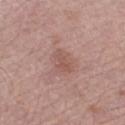biopsy status: imaged on a skin check; not biopsied
lesion size: about 3 mm
tile lighting: white-light illumination
image source: ~15 mm tile from a whole-body skin photo
body site: the left lower leg
automated lesion analysis: an outline eccentricity of about 0.85 (0 = round, 1 = elongated) and two-axis asymmetry of about 0.4; an average lesion color of about L≈53 a*≈22 b*≈24 (CIELAB), roughly 7 lightness units darker than nearby skin, and a normalized lesion–skin contrast near 5.5; border irregularity of about 4.5 on a 0–10 scale, internal color variation of about 1.5 on a 0–10 scale, and peripheral color asymmetry of about 0.5
subject: female, approximately 65 years of age